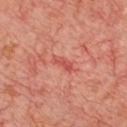anatomic site: the chest
patient: male, in their mid-60s
diameter: ~3 mm (longest diameter)
automated lesion analysis: a border-irregularity rating of about 3.5/10, a color-variation rating of about 0/10, and a peripheral color-asymmetry measure near 0
illumination: cross-polarized illumination
acquisition: total-body-photography crop, ~15 mm field of view